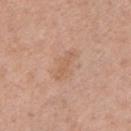Impression: No biopsy was performed on this lesion — it was imaged during a full skin examination and was not determined to be concerning. Background: Automated tile analysis of the lesion measured an area of roughly 5.5 mm², an eccentricity of roughly 0.9, and two-axis asymmetry of about 0.3. The analysis additionally found a border-irregularity index near 4/10, a color-variation rating of about 1.5/10, and peripheral color asymmetry of about 0.5. It also reported a classifier nevus-likeness of about 0/100 and lesion-presence confidence of about 100/100. A female patient, aged around 40. A region of skin cropped from a whole-body photographic capture, roughly 15 mm wide. This is a white-light tile. Located on the left upper arm.